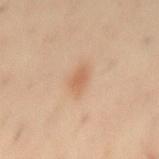  biopsy_status: not biopsied; imaged during a skin examination
  patient:
    sex: male
    age_approx: 40
  site: back
  lesion_size:
    long_diameter_mm_approx: 3.0
  image:
    source: total-body photography crop
    field_of_view_mm: 15
  lighting: cross-polarized